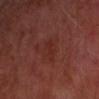Q: Was this lesion biopsied?
A: catalogued during a skin exam; not biopsied
Q: What kind of image is this?
A: ~15 mm tile from a whole-body skin photo
Q: Who is the patient?
A: male, aged around 50
Q: What did automated image analysis measure?
A: border irregularity of about 2 on a 0–10 scale, a color-variation rating of about 1.5/10, and radial color variation of about 0.5
Q: How was the tile lit?
A: cross-polarized
Q: Where on the body is the lesion?
A: the left forearm